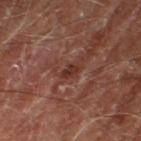biopsy_status: not biopsied; imaged during a skin examination
site: right thigh
lesion_size:
  long_diameter_mm_approx: 2.5
lighting: cross-polarized
patient:
  sex: male
  age_approx: 70
automated_metrics:
  cielab_L: 32
  cielab_a: 24
  cielab_b: 25
  vs_skin_darker_L: 8.0
  vs_skin_contrast_norm: 8.0
  peripheral_color_asymmetry: 0.0
  lesion_detection_confidence_0_100: 75
image:
  source: total-body photography crop
  field_of_view_mm: 15